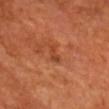notes: imaged on a skin check; not biopsied
subject: male, aged around 65
image: total-body-photography crop, ~15 mm field of view
anatomic site: the head or neck
diameter: about 2.5 mm